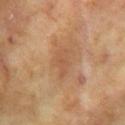  biopsy_status: not biopsied; imaged during a skin examination
  automated_metrics:
    area_mm2_approx: 5.0
    nevus_likeness_0_100: 0
    lesion_detection_confidence_0_100: 100
  patient:
    sex: female
    age_approx: 75
  lighting: cross-polarized
  site: arm
  image:
    source: total-body photography crop
    field_of_view_mm: 15